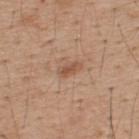The lesion was photographed on a routine skin check and not biopsied; there is no pathology result.
Cropped from a whole-body photographic skin survey; the tile spans about 15 mm.
A male patient, in their 40s.
An algorithmic analysis of the crop reported an area of roughly 3.5 mm² and an eccentricity of roughly 0.85.
This is a white-light tile.
The lesion is located on the back.